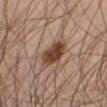The recorded lesion diameter is about 5 mm.
An algorithmic analysis of the crop reported a footprint of about 9.5 mm², an outline eccentricity of about 0.85 (0 = round, 1 = elongated), and two-axis asymmetry of about 0.25. The analysis additionally found an average lesion color of about L≈42 a*≈20 b*≈27 (CIELAB), a lesion–skin lightness drop of about 14, and a lesion-to-skin contrast of about 11 (normalized; higher = more distinct). The software also gave border irregularity of about 2.5 on a 0–10 scale and a within-lesion color-variation index near 5/10.
From the abdomen.
Imaged with cross-polarized lighting.
The patient is a male about 50 years old.
A roughly 15 mm field-of-view crop from a total-body skin photograph.